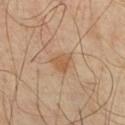Q: Was this lesion biopsied?
A: no biopsy performed (imaged during a skin exam)
Q: Patient demographics?
A: male, about 40 years old
Q: How was this image acquired?
A: total-body-photography crop, ~15 mm field of view
Q: What is the anatomic site?
A: the front of the torso
Q: What did automated image analysis measure?
A: a footprint of about 4.5 mm², an outline eccentricity of about 0.45 (0 = round, 1 = elongated), and a symmetry-axis asymmetry near 0.4; a mean CIELAB color near L≈56 a*≈19 b*≈35 and a normalized lesion–skin contrast near 6.5; a border-irregularity rating of about 4/10, internal color variation of about 2 on a 0–10 scale, and peripheral color asymmetry of about 0.5; an automated nevus-likeness rating near 50 out of 100 and a lesion-detection confidence of about 100/100
Q: What lighting was used for the tile?
A: cross-polarized